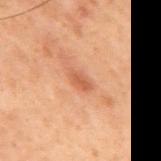Assessment:
The lesion was photographed on a routine skin check and not biopsied; there is no pathology result.
Acquisition and patient details:
The patient is a male aged around 70. On the mid back. A 15 mm crop from a total-body photograph taken for skin-cancer surveillance. Approximately 3 mm at its widest. The total-body-photography lesion software estimated a lesion area of about 4 mm², an outline eccentricity of about 0.85 (0 = round, 1 = elongated), and a symmetry-axis asymmetry near 0.25. It also reported a nevus-likeness score of about 10/100 and lesion-presence confidence of about 100/100. Imaged with cross-polarized lighting.